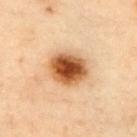workup = imaged on a skin check; not biopsied
image = total-body-photography crop, ~15 mm field of view
patient = male, about 55 years old
lighting = cross-polarized illumination
diameter = about 4.5 mm
site = the chest